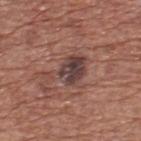biopsy status: imaged on a skin check; not biopsied | lesion diameter: about 5.5 mm | TBP lesion metrics: a footprint of about 10 mm² and a shape-asymmetry score of about 0.5 (0 = symmetric); an average lesion color of about L≈41 a*≈19 b*≈20 (CIELAB), a lesion–skin lightness drop of about 11, and a normalized lesion–skin contrast near 10; border irregularity of about 5.5 on a 0–10 scale, a color-variation rating of about 4/10, and radial color variation of about 1.5; a nevus-likeness score of about 5/100 and lesion-presence confidence of about 100/100 | subject: male, roughly 75 years of age | acquisition: total-body-photography crop, ~15 mm field of view | location: the upper back | lighting: white-light.